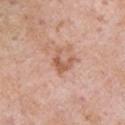{"biopsy_status": "not biopsied; imaged during a skin examination", "image": {"source": "total-body photography crop", "field_of_view_mm": 15}, "lighting": "white-light", "patient": {"sex": "male", "age_approx": 55}, "lesion_size": {"long_diameter_mm_approx": 3.0}, "site": "left upper arm"}